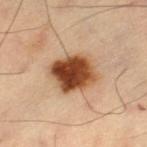Case summary:
– biopsy status: no biopsy performed (imaged during a skin exam)
– subject: male, about 60 years old
– size: ≈5 mm
– imaging modality: 15 mm crop, total-body photography
– illumination: cross-polarized
– automated metrics: border irregularity of about 1.5 on a 0–10 scale, internal color variation of about 9 on a 0–10 scale, and peripheral color asymmetry of about 3; an automated nevus-likeness rating near 100 out of 100 and a detector confidence of about 100 out of 100 that the crop contains a lesion
– location: the left thigh